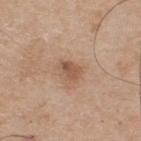image-analysis metrics: a lesion area of about 4.5 mm², an eccentricity of roughly 0.7, and a shape-asymmetry score of about 0.3 (0 = symmetric); a mean CIELAB color near L≈54 a*≈19 b*≈31 and about 10 CIELAB-L* units darker than the surrounding skin; internal color variation of about 3 on a 0–10 scale and radial color variation of about 1
patient: male, aged approximately 75
image source: 15 mm crop, total-body photography
lighting: white-light illumination
location: the upper back